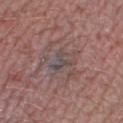biopsy_status: not biopsied; imaged during a skin examination
patient:
  sex: male
  age_approx: 45
image:
  source: total-body photography crop
  field_of_view_mm: 15
site: right lower leg
lighting: white-light